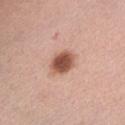Captured during whole-body skin photography for melanoma surveillance; the lesion was not biopsied. On the abdomen. A lesion tile, about 15 mm wide, cut from a 3D total-body photograph. A female subject about 60 years old.